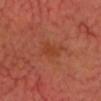The lesion was tiled from a total-body skin photograph and was not biopsied.
The tile uses cross-polarized illumination.
The patient is in their mid-60s.
From the head or neck.
The lesion-visualizer software estimated a lesion color around L≈43 a*≈28 b*≈34 in CIELAB, about 5 CIELAB-L* units darker than the surrounding skin, and a lesion-to-skin contrast of about 4.5 (normalized; higher = more distinct).
A 15 mm close-up tile from a total-body photography series done for melanoma screening.